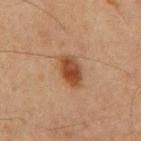Case summary:
* notes — no biopsy performed (imaged during a skin exam)
* site — the mid back
* image-analysis metrics — a nevus-likeness score of about 100/100 and a lesion-detection confidence of about 100/100
* subject — male, in their mid-60s
* image — ~15 mm tile from a whole-body skin photo